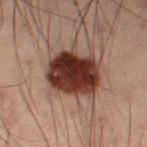lighting: cross-polarized
image:
  source: total-body photography crop
  field_of_view_mm: 15
site: left thigh
patient:
  sex: male
  age_approx: 55